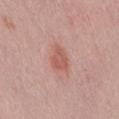{
  "biopsy_status": "not biopsied; imaged during a skin examination",
  "site": "lower back",
  "patient": {
    "sex": "male",
    "age_approx": 40
  },
  "lighting": "white-light",
  "image": {
    "source": "total-body photography crop",
    "field_of_view_mm": 15
  },
  "lesion_size": {
    "long_diameter_mm_approx": 3.0
  }
}